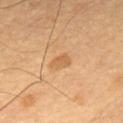Q: Was this lesion biopsied?
A: total-body-photography surveillance lesion; no biopsy
Q: Who is the patient?
A: male, in their mid-50s
Q: What is the imaging modality?
A: total-body-photography crop, ~15 mm field of view
Q: Lesion location?
A: the upper back